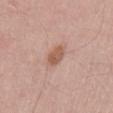Assessment: The lesion was photographed on a routine skin check and not biopsied; there is no pathology result. Acquisition and patient details: The lesion is located on the chest. The lesion's longest dimension is about 3 mm. The tile uses white-light illumination. A male patient approximately 65 years of age. A 15 mm crop from a total-body photograph taken for skin-cancer surveillance.